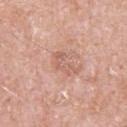Part of a total-body skin-imaging series; this lesion was reviewed on a skin check and was not flagged for biopsy. Longest diameter approximately 3.5 mm. A close-up tile cropped from a whole-body skin photograph, about 15 mm across. Captured under white-light illumination. Located on the arm. The subject is a male aged 78–82.